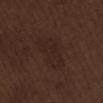| feature | finding |
|---|---|
| workup | total-body-photography surveillance lesion; no biopsy |
| body site | the abdomen |
| image-analysis metrics | a normalized border contrast of about 4.5; a border-irregularity rating of about 3/10 and radial color variation of about 0.5; a detector confidence of about 100 out of 100 that the crop contains a lesion |
| patient | male, aged 68–72 |
| tile lighting | white-light |
| acquisition | ~15 mm crop, total-body skin-cancer survey |
| diameter | about 6 mm |